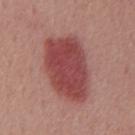Assessment: Captured during whole-body skin photography for melanoma surveillance; the lesion was not biopsied.